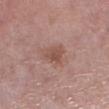Case summary:
- subject — female, aged 48–52
- location — the leg
- image — ~15 mm tile from a whole-body skin photo